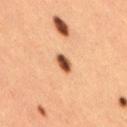The lesion was tiled from a total-body skin photograph and was not biopsied.
The tile uses cross-polarized illumination.
The patient is a female approximately 40 years of age.
A lesion tile, about 15 mm wide, cut from a 3D total-body photograph.
An algorithmic analysis of the crop reported an area of roughly 3.5 mm² and two-axis asymmetry of about 0.25. The analysis additionally found a border-irregularity index near 2/10, a color-variation rating of about 4/10, and a peripheral color-asymmetry measure near 1.5.
From the right thigh.
Approximately 2.5 mm at its widest.